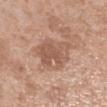No biopsy was performed on this lesion — it was imaged during a full skin examination and was not determined to be concerning. The lesion is on the right forearm. A female patient in their 50s. Automated tile analysis of the lesion measured a lesion–skin lightness drop of about 10 and a normalized border contrast of about 6.5. It also reported an automated nevus-likeness rating near 0 out of 100 and a detector confidence of about 100 out of 100 that the crop contains a lesion. Imaged with white-light lighting. A 15 mm crop from a total-body photograph taken for skin-cancer surveillance. About 6 mm across.